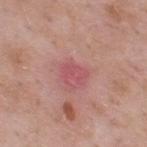This lesion was catalogued during total-body skin photography and was not selected for biopsy.
On the upper back.
A 15 mm crop from a total-body photograph taken for skin-cancer surveillance.
A male patient, roughly 55 years of age.
Approximately 2.5 mm at its widest.